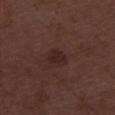Clinical summary: Captured under white-light illumination. Longest diameter approximately 2.5 mm. Automated tile analysis of the lesion measured an area of roughly 4 mm², a shape eccentricity near 0.7, and two-axis asymmetry of about 0.3. It also reported an average lesion color of about L≈23 a*≈19 b*≈20 (CIELAB). The software also gave internal color variation of about 1.5 on a 0–10 scale and radial color variation of about 0.5. A 15 mm close-up tile from a total-body photography series done for melanoma screening. A male patient aged around 50.